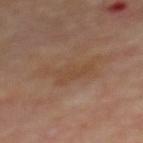{"biopsy_status": "not biopsied; imaged during a skin examination", "site": "mid back", "patient": {"sex": "male", "age_approx": 70}, "image": {"source": "total-body photography crop", "field_of_view_mm": 15}}